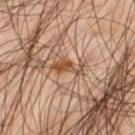Q: Is there a histopathology result?
A: no biopsy performed (imaged during a skin exam)
Q: How was the tile lit?
A: cross-polarized illumination
Q: Where on the body is the lesion?
A: the left thigh
Q: How was this image acquired?
A: ~15 mm tile from a whole-body skin photo
Q: Who is the patient?
A: male, in their mid- to late 40s